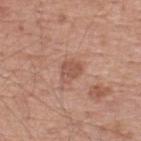workup: catalogued during a skin exam; not biopsied | image-analysis metrics: a mean CIELAB color near L≈54 a*≈22 b*≈29, a lesion–skin lightness drop of about 8, and a lesion-to-skin contrast of about 6 (normalized; higher = more distinct); a border-irregularity index near 2.5/10 and peripheral color asymmetry of about 1 | body site: the upper back | subject: male, aged around 55 | image source: ~15 mm tile from a whole-body skin photo.